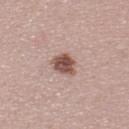<record>
<biopsy_status>not biopsied; imaged during a skin examination</biopsy_status>
<patient>
  <sex>male</sex>
  <age_approx>30</age_approx>
</patient>
<lesion_size>
  <long_diameter_mm_approx>3.0</long_diameter_mm_approx>
</lesion_size>
<image>
  <source>total-body photography crop</source>
  <field_of_view_mm>15</field_of_view_mm>
</image>
<lighting>white-light</lighting>
</record>